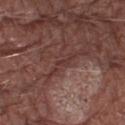Imaged during a routine full-body skin examination; the lesion was not biopsied and no histopathology is available.
The recorded lesion diameter is about 1.5 mm.
The lesion is on the left thigh.
The subject is a male aged 73–77.
A close-up tile cropped from a whole-body skin photograph, about 15 mm across.
Captured under white-light illumination.